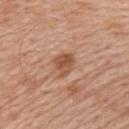Clinical impression: Captured during whole-body skin photography for melanoma surveillance; the lesion was not biopsied. Background: A 15 mm close-up tile from a total-body photography series done for melanoma screening. About 3 mm across. The lesion-visualizer software estimated a footprint of about 6 mm², an outline eccentricity of about 0.6 (0 = round, 1 = elongated), and a symmetry-axis asymmetry near 0.25. The software also gave a lesion color around L≈53 a*≈22 b*≈33 in CIELAB and roughly 10 lightness units darker than nearby skin. On the left upper arm. The subject is a male in their mid- to late 50s. Captured under white-light illumination.